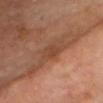Q: Is there a histopathology result?
A: no biopsy performed (imaged during a skin exam)
Q: What is the anatomic site?
A: the head or neck
Q: What kind of image is this?
A: ~15 mm tile from a whole-body skin photo
Q: What are the patient's age and sex?
A: male, in their 70s